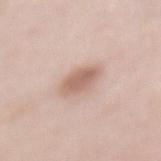  biopsy_status: not biopsied; imaged during a skin examination
  image:
    source: total-body photography crop
    field_of_view_mm: 15
  site: mid back
  patient:
    sex: female
    age_approx: 50